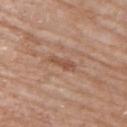Captured during whole-body skin photography for melanoma surveillance; the lesion was not biopsied. This is a white-light tile. Approximately 3.5 mm at its widest. A roughly 15 mm field-of-view crop from a total-body skin photograph. A female subject, aged approximately 75. Located on the upper back.